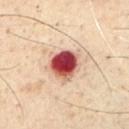<tbp_lesion>
<biopsy_status>not biopsied; imaged during a skin examination</biopsy_status>
<image>
  <source>total-body photography crop</source>
  <field_of_view_mm>15</field_of_view_mm>
</image>
<site>chest</site>
<patient>
  <sex>male</sex>
  <age_approx>70</age_approx>
</patient>
</tbp_lesion>